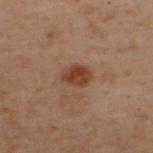<case>
<biopsy_status>not biopsied; imaged during a skin examination</biopsy_status>
<lesion_size>
  <long_diameter_mm_approx>3.5</long_diameter_mm_approx>
</lesion_size>
<site>upper back</site>
<image>
  <source>total-body photography crop</source>
  <field_of_view_mm>15</field_of_view_mm>
</image>
<lighting>cross-polarized</lighting>
<automated_metrics>
  <area_mm2_approx>6.0</area_mm2_approx>
  <shape_asymmetry>0.15</shape_asymmetry>
</automated_metrics>
<patient>
  <sex>male</sex>
  <age_approx>50</age_approx>
</patient>
</case>